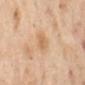Part of a total-body skin-imaging series; this lesion was reviewed on a skin check and was not flagged for biopsy.
An algorithmic analysis of the crop reported a footprint of about 4 mm², a shape eccentricity near 0.7, and two-axis asymmetry of about 0.25. The software also gave a normalized lesion–skin contrast near 5.5. It also reported border irregularity of about 2.5 on a 0–10 scale and peripheral color asymmetry of about 0.5.
The lesion is located on the mid back.
A female patient, aged 53–57.
A region of skin cropped from a whole-body photographic capture, roughly 15 mm wide.
Imaged with cross-polarized lighting.
Approximately 3 mm at its widest.